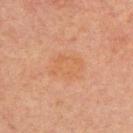– workup · no biopsy performed (imaged during a skin exam)
– subject · male, aged 28–32
– anatomic site · the upper back
– acquisition · ~15 mm tile from a whole-body skin photo